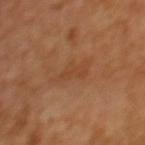  biopsy_status: not biopsied; imaged during a skin examination
  patient:
    sex: female
    age_approx: 40
  image:
    source: total-body photography crop
    field_of_view_mm: 15
  site: upper back